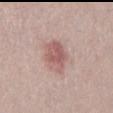Assessment: This lesion was catalogued during total-body skin photography and was not selected for biopsy. Acquisition and patient details: A 15 mm close-up extracted from a 3D total-body photography capture. A male subject, about 30 years old. Measured at roughly 4.5 mm in maximum diameter.